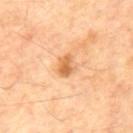<lesion>
<biopsy_status>not biopsied; imaged during a skin examination</biopsy_status>
<automated_metrics>
  <vs_skin_darker_L>12.0</vs_skin_darker_L>
  <vs_skin_contrast_norm>8.0</vs_skin_contrast_norm>
  <border_irregularity_0_10>2.5</border_irregularity_0_10>
  <color_variation_0_10>3.5</color_variation_0_10>
  <peripheral_color_asymmetry>1.5</peripheral_color_asymmetry>
</automated_metrics>
<lighting>cross-polarized</lighting>
<image>
  <source>total-body photography crop</source>
  <field_of_view_mm>15</field_of_view_mm>
</image>
<site>mid back</site>
<patient>
  <sex>male</sex>
  <age_approx>70</age_approx>
</patient>
</lesion>